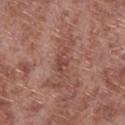Case summary:
• notes: no biopsy performed (imaged during a skin exam)
• subject: male, aged 53 to 57
• anatomic site: the leg
• acquisition: 15 mm crop, total-body photography
• illumination: white-light
• size: about 3.5 mm